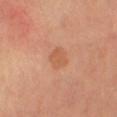{
  "biopsy_status": "not biopsied; imaged during a skin examination",
  "lighting": "cross-polarized",
  "patient": {
    "sex": "female",
    "age_approx": 65
  },
  "lesion_size": {
    "long_diameter_mm_approx": 3.0
  },
  "site": "left lower leg",
  "image": {
    "source": "total-body photography crop",
    "field_of_view_mm": 15
  },
  "automated_metrics": {
    "area_mm2_approx": 4.5,
    "shape_asymmetry": 0.2
  }
}